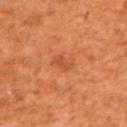{"patient": {"sex": "male", "age_approx": 65}, "lighting": "cross-polarized", "automated_metrics": {"cielab_L": 49, "cielab_a": 31, "cielab_b": 40, "vs_skin_darker_L": 7.0, "vs_skin_contrast_norm": 5.5, "border_irregularity_0_10": 4.0, "color_variation_0_10": 0.0, "peripheral_color_asymmetry": 0.0, "nevus_likeness_0_100": 0, "lesion_detection_confidence_0_100": 100}, "lesion_size": {"long_diameter_mm_approx": 2.5}, "site": "back", "image": {"source": "total-body photography crop", "field_of_view_mm": 15}}